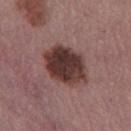The lesion was tiled from a total-body skin photograph and was not biopsied. A female subject aged 53–57. This is a white-light tile. Located on the left thigh. Cropped from a total-body skin-imaging series; the visible field is about 15 mm.